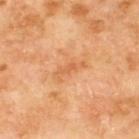<record>
  <patient>
    <sex>male</sex>
    <age_approx>70</age_approx>
  </patient>
  <automated_metrics>
    <border_irregularity_0_10>6.5</border_irregularity_0_10>
    <color_variation_0_10>0.0</color_variation_0_10>
    <peripheral_color_asymmetry>0.0</peripheral_color_asymmetry>
  </automated_metrics>
  <site>upper back</site>
  <lesion_size>
    <long_diameter_mm_approx>3.5</long_diameter_mm_approx>
  </lesion_size>
  <lighting>cross-polarized</lighting>
  <image>
    <source>total-body photography crop</source>
    <field_of_view_mm>15</field_of_view_mm>
  </image>
</record>